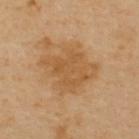image source = 15 mm crop, total-body photography; patient = male, in their mid-50s; anatomic site = the upper back.